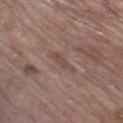Q: Patient demographics?
A: female, aged 68 to 72
Q: Lesion size?
A: ~3 mm (longest diameter)
Q: What is the imaging modality?
A: ~15 mm crop, total-body skin-cancer survey
Q: Lesion location?
A: the right thigh
Q: How was the tile lit?
A: white-light illumination
Q: Automated lesion metrics?
A: two-axis asymmetry of about 0.35; a mean CIELAB color near L≈47 a*≈18 b*≈23, about 6 CIELAB-L* units darker than the surrounding skin, and a normalized border contrast of about 5.5; a border-irregularity rating of about 4.5/10, a color-variation rating of about 0.5/10, and a peripheral color-asymmetry measure near 0; a lesion-detection confidence of about 100/100